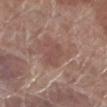  biopsy_status: not biopsied; imaged during a skin examination
  patient:
    sex: male
    age_approx: 70
  site: left lower leg
  lighting: white-light
  automated_metrics:
    area_mm2_approx: 6.0
    eccentricity: 0.8
    shape_asymmetry: 0.25
    vs_skin_darker_L: 6.0
    vs_skin_contrast_norm: 5.0
    border_irregularity_0_10: 3.5
    peripheral_color_asymmetry: 0.5
    nevus_likeness_0_100: 0
    lesion_detection_confidence_0_100: 100
  lesion_size:
    long_diameter_mm_approx: 3.5
  image:
    source: total-body photography crop
    field_of_view_mm: 15